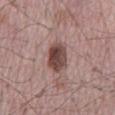notes: imaged on a skin check; not biopsied
subject: male, in their mid- to late 60s
automated lesion analysis: a footprint of about 9.5 mm² and an outline eccentricity of about 0.85 (0 = round, 1 = elongated)
anatomic site: the mid back
tile lighting: white-light
lesion size: ≈4.5 mm
image: total-body-photography crop, ~15 mm field of view